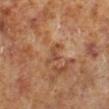The lesion was tiled from a total-body skin photograph and was not biopsied. Located on the left lower leg. The patient is a male roughly 60 years of age. A 15 mm close-up tile from a total-body photography series done for melanoma screening. The lesion's longest dimension is about 3 mm. This is a cross-polarized tile.